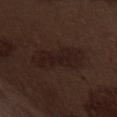<lesion>
<biopsy_status>not biopsied; imaged during a skin examination</biopsy_status>
<lighting>white-light</lighting>
<patient>
  <sex>male</sex>
  <age_approx>70</age_approx>
</patient>
<site>abdomen</site>
<lesion_size>
  <long_diameter_mm_approx>7.0</long_diameter_mm_approx>
</lesion_size>
<image>
  <source>total-body photography crop</source>
  <field_of_view_mm>15</field_of_view_mm>
</image>
</lesion>